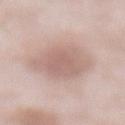Impression: Imaged during a routine full-body skin examination; the lesion was not biopsied and no histopathology is available. Context: The total-body-photography lesion software estimated a lesion area of about 21 mm², a shape eccentricity near 0.55, and two-axis asymmetry of about 0.15. It also reported an average lesion color of about L≈63 a*≈18 b*≈23 (CIELAB) and a normalized lesion–skin contrast near 6. And it measured border irregularity of about 2 on a 0–10 scale, a color-variation rating of about 2.5/10, and peripheral color asymmetry of about 0.5. The software also gave an automated nevus-likeness rating near 25 out of 100. The subject is a male in their mid- to late 50s. Imaged with white-light lighting. On the abdomen. A 15 mm close-up extracted from a 3D total-body photography capture.